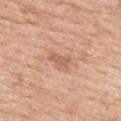follow-up = no biopsy performed (imaged during a skin exam); acquisition = total-body-photography crop, ~15 mm field of view; location = the upper back; patient = female, aged approximately 55.